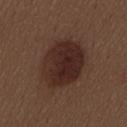workup = no biopsy performed (imaged during a skin exam)
lesion diameter = about 6.5 mm
subject = female, about 30 years old
site = the mid back
imaging modality = total-body-photography crop, ~15 mm field of view